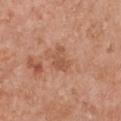The lesion was tiled from a total-body skin photograph and was not biopsied.
A female subject approximately 60 years of age.
Longest diameter approximately 3.5 mm.
A close-up tile cropped from a whole-body skin photograph, about 15 mm across.
Located on the chest.